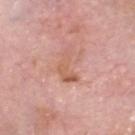| feature | finding |
|---|---|
| workup | no biopsy performed (imaged during a skin exam) |
| body site | the head or neck |
| size | about 4 mm |
| image | ~15 mm crop, total-body skin-cancer survey |
| patient | male, roughly 60 years of age |
| lighting | white-light |
| automated metrics | border irregularity of about 6 on a 0–10 scale and a within-lesion color-variation index near 4/10 |